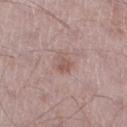biopsy_status: not biopsied; imaged during a skin examination
image:
  source: total-body photography crop
  field_of_view_mm: 15
patient:
  sex: male
  age_approx: 50
lighting: white-light
site: right thigh
automated_metrics:
  area_mm2_approx: 4.0
  eccentricity: 0.7
  cielab_L: 55
  cielab_a: 18
  cielab_b: 23
  vs_skin_contrast_norm: 6.0
  nevus_likeness_0_100: 5
  lesion_detection_confidence_0_100: 100